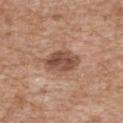Part of a total-body skin-imaging series; this lesion was reviewed on a skin check and was not flagged for biopsy. The subject is a male about 60 years old. Longest diameter approximately 4.5 mm. From the chest. Captured under white-light illumination. A 15 mm close-up extracted from a 3D total-body photography capture. The lesion-visualizer software estimated border irregularity of about 1.5 on a 0–10 scale and a color-variation rating of about 4.5/10.